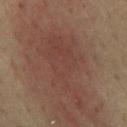Notes:
• follow-up · total-body-photography surveillance lesion; no biopsy
• patient · male, roughly 65 years of age
• lighting · cross-polarized illumination
• acquisition · ~15 mm tile from a whole-body skin photo
• anatomic site · the mid back